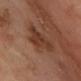This lesion was catalogued during total-body skin photography and was not selected for biopsy. A roughly 15 mm field-of-view crop from a total-body skin photograph. A male patient, roughly 70 years of age. The tile uses cross-polarized illumination. The lesion's longest dimension is about 5.5 mm. The total-body-photography lesion software estimated an area of roughly 12 mm² and two-axis asymmetry of about 0.4. And it measured a mean CIELAB color near L≈36 a*≈21 b*≈28, roughly 8 lightness units darker than nearby skin, and a lesion-to-skin contrast of about 7.5 (normalized; higher = more distinct). The software also gave a classifier nevus-likeness of about 10/100. Located on the chest.